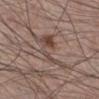The lesion was tiled from a total-body skin photograph and was not biopsied. Longest diameter approximately 5 mm. A close-up tile cropped from a whole-body skin photograph, about 15 mm across. The tile uses white-light illumination. The lesion is on the right lower leg. A male patient, aged around 60.